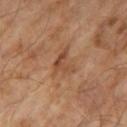<lesion>
  <biopsy_status>not biopsied; imaged during a skin examination</biopsy_status>
  <patient>
    <sex>male</sex>
    <age_approx>65</age_approx>
  </patient>
  <image>
    <source>total-body photography crop</source>
    <field_of_view_mm>15</field_of_view_mm>
  </image>
  <automated_metrics>
    <area_mm2_approx>5.0</area_mm2_approx>
    <eccentricity>0.65</eccentricity>
    <shape_asymmetry>0.55</shape_asymmetry>
    <cielab_L>46</cielab_L>
    <cielab_a>21</cielab_a>
    <cielab_b>32</cielab_b>
    <vs_skin_darker_L>8.0</vs_skin_darker_L>
    <vs_skin_contrast_norm>6.0</vs_skin_contrast_norm>
    <nevus_likeness_0_100>0</nevus_likeness_0_100>
  </automated_metrics>
</lesion>